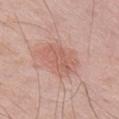biopsy status: imaged on a skin check; not biopsied | lighting: white-light | anatomic site: the right upper arm | imaging modality: total-body-photography crop, ~15 mm field of view | subject: male, roughly 65 years of age.